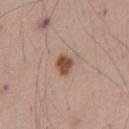The lesion was tiled from a total-body skin photograph and was not biopsied. This image is a 15 mm lesion crop taken from a total-body photograph. The lesion is on the abdomen. The lesion-visualizer software estimated an eccentricity of roughly 0.5. And it measured an automated nevus-likeness rating near 95 out of 100 and lesion-presence confidence of about 100/100. Captured under white-light illumination. A male subject aged around 40. Longest diameter approximately 2.5 mm.